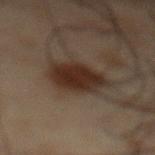No biopsy was performed on this lesion — it was imaged during a full skin examination and was not determined to be concerning. On the abdomen. A male subject, aged approximately 50. Cropped from a total-body skin-imaging series; the visible field is about 15 mm.